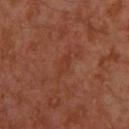Case summary:
- follow-up: catalogued during a skin exam; not biopsied